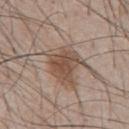follow-up = imaged on a skin check; not biopsied
subject = male, in their mid-40s
imaging modality = total-body-photography crop, ~15 mm field of view
location = the front of the torso
illumination = white-light